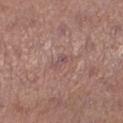workup: total-body-photography surveillance lesion; no biopsy
size: ~2.5 mm (longest diameter)
acquisition: 15 mm crop, total-body photography
body site: the leg
TBP lesion metrics: a footprint of about 2.5 mm² and an outline eccentricity of about 0.9 (0 = round, 1 = elongated); a mean CIELAB color near L≈50 a*≈20 b*≈19, a lesion–skin lightness drop of about 7, and a normalized lesion–skin contrast near 5.5; a border-irregularity index near 5/10, internal color variation of about 0 on a 0–10 scale, and radial color variation of about 0; an automated nevus-likeness rating near 0 out of 100 and a detector confidence of about 80 out of 100 that the crop contains a lesion
tile lighting: white-light
subject: female, aged around 50